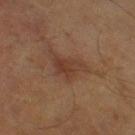No biopsy was performed on this lesion — it was imaged during a full skin examination and was not determined to be concerning. The lesion is on the left leg. Measured at roughly 4 mm in maximum diameter. Imaged with cross-polarized lighting. This image is a 15 mm lesion crop taken from a total-body photograph. Automated image analysis of the tile measured an automated nevus-likeness rating near 0 out of 100 and a lesion-detection confidence of about 100/100. A male subject, in their mid- to late 60s.